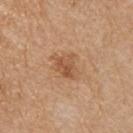Impression: This lesion was catalogued during total-body skin photography and was not selected for biopsy. Background: Imaged with white-light lighting. A region of skin cropped from a whole-body photographic capture, roughly 15 mm wide. The lesion is located on the front of the torso. The lesion-visualizer software estimated radial color variation of about 1.5. The subject is a male aged 68 to 72.